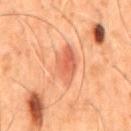  biopsy_status: not biopsied; imaged during a skin examination
  image:
    source: total-body photography crop
    field_of_view_mm: 15
  lesion_size:
    long_diameter_mm_approx: 5.5
  site: mid back
  automated_metrics:
    cielab_L: 49
    cielab_a: 24
    cielab_b: 31
    vs_skin_darker_L: 7.0
    border_irregularity_0_10: 3.0
    color_variation_0_10: 5.0
    lesion_detection_confidence_0_100: 100
  lighting: cross-polarized
  patient:
    sex: male
    age_approx: 50